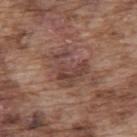Notes:
– follow-up — catalogued during a skin exam; not biopsied
– image source — total-body-photography crop, ~15 mm field of view
– patient — male, in their mid-70s
– location — the upper back